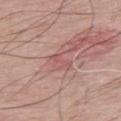Notes:
- patient — male, aged approximately 65
- body site — the upper back
- imaging modality — total-body-photography crop, ~15 mm field of view
- automated metrics — an outline eccentricity of about 0.95 (0 = round, 1 = elongated) and a shape-asymmetry score of about 0.4 (0 = symmetric); an average lesion color of about L≈55 a*≈24 b*≈24 (CIELAB) and about 6 CIELAB-L* units darker than the surrounding skin; a border-irregularity rating of about 5/10, a within-lesion color-variation index near 0/10, and peripheral color asymmetry of about 0; a classifier nevus-likeness of about 0/100 and lesion-presence confidence of about 80/100
- lesion size — ~3 mm (longest diameter)